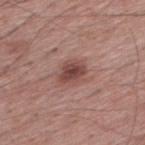patient: male, aged 53–57; image source: ~15 mm crop, total-body skin-cancer survey; location: the upper back.